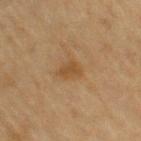Clinical summary:
Captured under cross-polarized illumination. A female subject aged 78 to 82. Automated image analysis of the tile measured a lesion area of about 4.5 mm², an eccentricity of roughly 0.7, and two-axis asymmetry of about 0.4. The lesion is located on the right upper arm. The lesion's longest dimension is about 3 mm. A roughly 15 mm field-of-view crop from a total-body skin photograph.